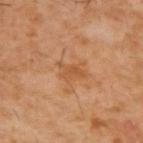Q: Lesion size?
A: ~3.5 mm (longest diameter)
Q: What did automated image analysis measure?
A: a lesion area of about 4.5 mm², an outline eccentricity of about 0.8 (0 = round, 1 = elongated), and two-axis asymmetry of about 0.6; a border-irregularity rating of about 6.5/10, a within-lesion color-variation index near 1.5/10, and a peripheral color-asymmetry measure near 0.5
Q: What kind of image is this?
A: total-body-photography crop, ~15 mm field of view
Q: Who is the patient?
A: male, approximately 60 years of age
Q: What is the anatomic site?
A: the upper back